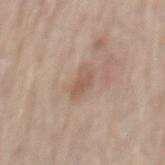<tbp_lesion>
  <biopsy_status>not biopsied; imaged during a skin examination</biopsy_status>
  <automated_metrics>
    <area_mm2_approx>5.0</area_mm2_approx>
    <eccentricity>0.8</eccentricity>
    <shape_asymmetry>0.3</shape_asymmetry>
  </automated_metrics>
  <patient>
    <sex>male</sex>
    <age_approx>80</age_approx>
  </patient>
  <image>
    <source>total-body photography crop</source>
    <field_of_view_mm>15</field_of_view_mm>
  </image>
  <site>mid back</site>
  <lesion_size>
    <long_diameter_mm_approx>3.0</long_diameter_mm_approx>
  </lesion_size>
</tbp_lesion>